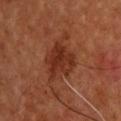This lesion was catalogued during total-body skin photography and was not selected for biopsy.
On the upper back.
A region of skin cropped from a whole-body photographic capture, roughly 15 mm wide.
A male patient, about 60 years old.